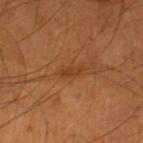No biopsy was performed on this lesion — it was imaged during a full skin examination and was not determined to be concerning. Cropped from a total-body skin-imaging series; the visible field is about 15 mm. The lesion's longest dimension is about 2.5 mm. A male patient, aged 53–57. Imaged with cross-polarized lighting. The total-body-photography lesion software estimated an average lesion color of about L≈38 a*≈24 b*≈35 (CIELAB), a lesion–skin lightness drop of about 7, and a lesion-to-skin contrast of about 6 (normalized; higher = more distinct). And it measured a border-irregularity rating of about 3/10, a within-lesion color-variation index near 0/10, and peripheral color asymmetry of about 0. And it measured a nevus-likeness score of about 5/100 and lesion-presence confidence of about 100/100. From the right lower leg.